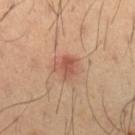Clinical impression: The lesion was tiled from a total-body skin photograph and was not biopsied. Acquisition and patient details: The lesion is on the left forearm. The lesion's longest dimension is about 3 mm. The subject is a male approximately 40 years of age. Cropped from a whole-body photographic skin survey; the tile spans about 15 mm. The tile uses cross-polarized illumination.